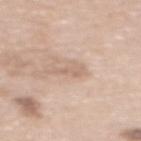Assessment: The lesion was tiled from a total-body skin photograph and was not biopsied. Acquisition and patient details: A female subject approximately 60 years of age. The lesion is located on the upper back. Cropped from a whole-body photographic skin survey; the tile spans about 15 mm. An algorithmic analysis of the crop reported a color-variation rating of about 1/10 and peripheral color asymmetry of about 0.5.